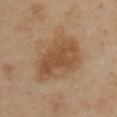Notes:
• workup · catalogued during a skin exam; not biopsied
• location · the chest
• imaging modality · ~15 mm crop, total-body skin-cancer survey
• patient · female, aged 38–42
• automated lesion analysis · a lesion area of about 21 mm² and an outline eccentricity of about 0.75 (0 = round, 1 = elongated); border irregularity of about 3 on a 0–10 scale, a within-lesion color-variation index near 3/10, and peripheral color asymmetry of about 1; lesion-presence confidence of about 100/100
• lighting · cross-polarized illumination